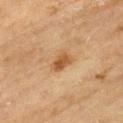notes = no biopsy performed (imaged during a skin exam)
lighting = cross-polarized
anatomic site = the arm
size = ~3 mm (longest diameter)
patient = male, aged 68–72
acquisition = ~15 mm crop, total-body skin-cancer survey